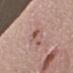| feature | finding |
|---|---|
| follow-up | total-body-photography surveillance lesion; no biopsy |
| subject | male, aged 63 to 67 |
| acquisition | 15 mm crop, total-body photography |
| illumination | white-light |
| body site | the right forearm |
| lesion size | ~4.5 mm (longest diameter) |
| automated lesion analysis | an area of roughly 5.5 mm², an outline eccentricity of about 0.95 (0 = round, 1 = elongated), and a shape-asymmetry score of about 0.4 (0 = symmetric); an average lesion color of about L≈55 a*≈21 b*≈25 (CIELAB), roughly 9 lightness units darker than nearby skin, and a normalized border contrast of about 6; a border-irregularity index near 7/10, internal color variation of about 2.5 on a 0–10 scale, and peripheral color asymmetry of about 0.5; an automated nevus-likeness rating near 0 out of 100 and a detector confidence of about 100 out of 100 that the crop contains a lesion |